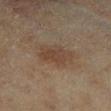Clinical impression: No biopsy was performed on this lesion — it was imaged during a full skin examination and was not determined to be concerning. Background: The recorded lesion diameter is about 4 mm. On the right lower leg. A close-up tile cropped from a whole-body skin photograph, about 15 mm across. A female subject roughly 60 years of age. The total-body-photography lesion software estimated a lesion area of about 11 mm², a shape eccentricity near 0.65, and two-axis asymmetry of about 0.2. The software also gave an average lesion color of about L≈38 a*≈14 b*≈25 (CIELAB) and about 6 CIELAB-L* units darker than the surrounding skin. It also reported an automated nevus-likeness rating near 35 out of 100 and lesion-presence confidence of about 100/100. The tile uses cross-polarized illumination.